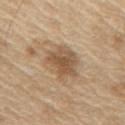Recorded during total-body skin imaging; not selected for excision or biopsy.